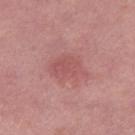<lesion>
  <biopsy_status>not biopsied; imaged during a skin examination</biopsy_status>
  <patient>
    <sex>female</sex>
    <age_approx>40</age_approx>
  </patient>
  <image>
    <source>total-body photography crop</source>
    <field_of_view_mm>15</field_of_view_mm>
  </image>
  <site>left thigh</site>
</lesion>